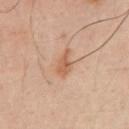follow-up = imaged on a skin check; not biopsied
size = ≈3.5 mm
patient = male, in their 40s
anatomic site = the arm
acquisition = ~15 mm tile from a whole-body skin photo
lighting = cross-polarized
automated lesion analysis = a mean CIELAB color near L≈57 a*≈21 b*≈33 and a normalized lesion–skin contrast near 7; a border-irregularity rating of about 4/10, a within-lesion color-variation index near 2/10, and peripheral color asymmetry of about 0.5; a nevus-likeness score of about 45/100 and a detector confidence of about 100 out of 100 that the crop contains a lesion